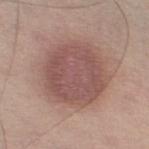Part of a total-body skin-imaging series; this lesion was reviewed on a skin check and was not flagged for biopsy. The lesion-visualizer software estimated an area of roughly 36 mm², an outline eccentricity of about 0.45 (0 = round, 1 = elongated), and a symmetry-axis asymmetry near 0.1. And it measured a lesion color around L≈52 a*≈20 b*≈22 in CIELAB, roughly 10 lightness units darker than nearby skin, and a normalized lesion–skin contrast near 7.5. The analysis additionally found a border-irregularity rating of about 1.5/10, a color-variation rating of about 3/10, and peripheral color asymmetry of about 1. It also reported a detector confidence of about 100 out of 100 that the crop contains a lesion. From the left thigh. The lesion's longest dimension is about 7 mm. This image is a 15 mm lesion crop taken from a total-body photograph. The patient is a male aged 38 to 42. Imaged with white-light lighting.